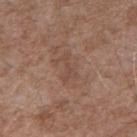Case summary:
- follow-up — no biopsy performed (imaged during a skin exam)
- patient — male, in their 50s
- location — the right upper arm
- acquisition — total-body-photography crop, ~15 mm field of view